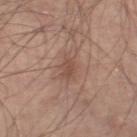body site = the right lower leg; size = ~3 mm (longest diameter); subject = male, roughly 60 years of age; acquisition = total-body-photography crop, ~15 mm field of view.